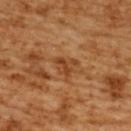Imaged during a routine full-body skin examination; the lesion was not biopsied and no histopathology is available.
A 15 mm crop from a total-body photograph taken for skin-cancer surveillance.
The lesion is on the upper back.
A female subject, in their mid- to late 50s.
The lesion's longest dimension is about 3 mm.